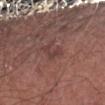Captured during whole-body skin photography for melanoma surveillance; the lesion was not biopsied.
A close-up tile cropped from a whole-body skin photograph, about 15 mm across.
A male subject, approximately 65 years of age.
The lesion is on the arm.